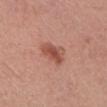This lesion was catalogued during total-body skin photography and was not selected for biopsy. Automated image analysis of the tile measured about 11 CIELAB-L* units darker than the surrounding skin. The software also gave border irregularity of about 3 on a 0–10 scale, a color-variation rating of about 3.5/10, and a peripheral color-asymmetry measure near 1. The software also gave lesion-presence confidence of about 100/100. This is a white-light tile. A female subject aged 23 to 27. The lesion is located on the left lower leg. A 15 mm close-up tile from a total-body photography series done for melanoma screening. The recorded lesion diameter is about 3.5 mm.